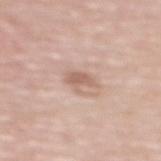| key | value |
|---|---|
| anatomic site | the front of the torso |
| lighting | white-light |
| image-analysis metrics | a footprint of about 4 mm², an outline eccentricity of about 0.7 (0 = round, 1 = elongated), and a symmetry-axis asymmetry near 0.4; border irregularity of about 4.5 on a 0–10 scale and radial color variation of about 0.5 |
| diameter | ≈2.5 mm |
| patient | female, aged 63 to 67 |
| image | ~15 mm tile from a whole-body skin photo |